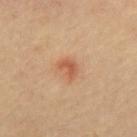workup = no biopsy performed (imaged during a skin exam) | lesion size = ≈2.5 mm | automated lesion analysis = a footprint of about 3.5 mm², an eccentricity of roughly 0.7, and two-axis asymmetry of about 0.4; roughly 9 lightness units darker than nearby skin and a normalized border contrast of about 6.5; an automated nevus-likeness rating near 35 out of 100 and a lesion-detection confidence of about 100/100 | acquisition = total-body-photography crop, ~15 mm field of view | lighting = cross-polarized | body site = the back | patient = male, roughly 65 years of age.